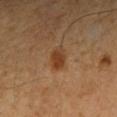follow-up: total-body-photography surveillance lesion; no biopsy
diameter: ~2.5 mm (longest diameter)
acquisition: ~15 mm crop, total-body skin-cancer survey
location: the left forearm
subject: female, roughly 50 years of age